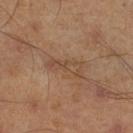follow-up — no biopsy performed (imaged during a skin exam)
diameter — ~4.5 mm (longest diameter)
imaging modality — ~15 mm tile from a whole-body skin photo
tile lighting — cross-polarized
body site — the leg
subject — male, in their mid-40s